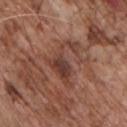Clinical impression:
No biopsy was performed on this lesion — it was imaged during a full skin examination and was not determined to be concerning.
Context:
Approximately 5.5 mm at its widest. This image is a 15 mm lesion crop taken from a total-body photograph. A male patient approximately 70 years of age. On the chest. The tile uses white-light illumination.